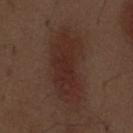Q: Was this lesion biopsied?
A: total-body-photography surveillance lesion; no biopsy
Q: What lighting was used for the tile?
A: white-light illumination
Q: Where on the body is the lesion?
A: the front of the torso
Q: What kind of image is this?
A: ~15 mm crop, total-body skin-cancer survey
Q: Automated lesion metrics?
A: an average lesion color of about L≈28 a*≈19 b*≈22 (CIELAB) and a normalized lesion–skin contrast near 7.5; a color-variation rating of about 2.5/10 and peripheral color asymmetry of about 1; an automated nevus-likeness rating near 100 out of 100 and a detector confidence of about 100 out of 100 that the crop contains a lesion
Q: Who is the patient?
A: male, about 70 years old